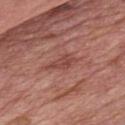No biopsy was performed on this lesion — it was imaged during a full skin examination and was not determined to be concerning.
Measured at roughly 4 mm in maximum diameter.
A male patient aged 58 to 62.
On the chest.
A lesion tile, about 15 mm wide, cut from a 3D total-body photograph.
The tile uses white-light illumination.